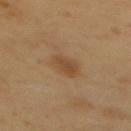| feature | finding |
|---|---|
| follow-up | catalogued during a skin exam; not biopsied |
| patient | female, aged around 55 |
| image source | 15 mm crop, total-body photography |
| anatomic site | the back |